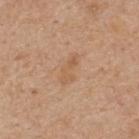  biopsy_status: not biopsied; imaged during a skin examination
  image:
    source: total-body photography crop
    field_of_view_mm: 15
  automated_metrics:
    border_irregularity_0_10: 4.0
    color_variation_0_10: 2.0
    peripheral_color_asymmetry: 0.5
  lesion_size:
    long_diameter_mm_approx: 4.0
  patient:
    sex: male
    age_approx: 65
  site: upper back